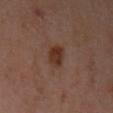Q: How large is the lesion?
A: ≈3.5 mm
Q: Automated lesion metrics?
A: a color-variation rating of about 2.5/10 and a peripheral color-asymmetry measure near 1; a nevus-likeness score of about 95/100 and a lesion-detection confidence of about 100/100
Q: Where on the body is the lesion?
A: the left upper arm
Q: How was the tile lit?
A: cross-polarized illumination
Q: Who is the patient?
A: female, approximately 40 years of age
Q: How was this image acquired?
A: total-body-photography crop, ~15 mm field of view